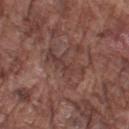| key | value |
|---|---|
| workup | catalogued during a skin exam; not biopsied |
| tile lighting | white-light illumination |
| image | 15 mm crop, total-body photography |
| automated metrics | a footprint of about 7.5 mm², an outline eccentricity of about 0.95 (0 = round, 1 = elongated), and a shape-asymmetry score of about 0.4 (0 = symmetric); a lesion–skin lightness drop of about 7 and a normalized lesion–skin contrast near 6; a border-irregularity rating of about 6.5/10, a within-lesion color-variation index near 3.5/10, and peripheral color asymmetry of about 1; a nevus-likeness score of about 0/100 and a lesion-detection confidence of about 55/100 |
| patient | male, in their mid- to late 70s |
| location | the left upper arm |